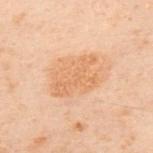{"biopsy_status": "not biopsied; imaged during a skin examination", "image": {"source": "total-body photography crop", "field_of_view_mm": 15}, "site": "back", "patient": {"sex": "male", "age_approx": 50}}